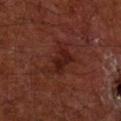{"biopsy_status": "not biopsied; imaged during a skin examination", "site": "left arm", "patient": {"sex": "male", "age_approx": 65}, "lesion_size": {"long_diameter_mm_approx": 5.0}, "automated_metrics": {"area_mm2_approx": 9.5, "eccentricity": 0.85, "border_irregularity_0_10": 6.5, "peripheral_color_asymmetry": 1.0, "nevus_likeness_0_100": 95, "lesion_detection_confidence_0_100": 100}, "lighting": "cross-polarized", "image": {"source": "total-body photography crop", "field_of_view_mm": 15}}